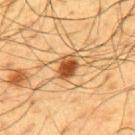Q: Is there a histopathology result?
A: total-body-photography surveillance lesion; no biopsy
Q: What did automated image analysis measure?
A: a footprint of about 6 mm², an outline eccentricity of about 0.65 (0 = round, 1 = elongated), and two-axis asymmetry of about 0.2; an average lesion color of about L≈41 a*≈20 b*≈34 (CIELAB) and a lesion–skin lightness drop of about 14; a border-irregularity index near 2/10, a within-lesion color-variation index near 5.5/10, and peripheral color asymmetry of about 1.5; a nevus-likeness score of about 100/100 and a lesion-detection confidence of about 100/100
Q: What are the patient's age and sex?
A: male, aged approximately 60
Q: What kind of image is this?
A: ~15 mm crop, total-body skin-cancer survey
Q: How was the tile lit?
A: cross-polarized
Q: Lesion location?
A: the upper back